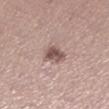Q: How was the tile lit?
A: white-light
Q: Patient demographics?
A: female, about 35 years old
Q: How large is the lesion?
A: about 3 mm
Q: What kind of image is this?
A: ~15 mm tile from a whole-body skin photo
Q: Where on the body is the lesion?
A: the left lower leg